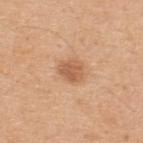The lesion was photographed on a routine skin check and not biopsied; there is no pathology result. Measured at roughly 3 mm in maximum diameter. A 15 mm close-up tile from a total-body photography series done for melanoma screening. Located on the upper back. A male subject roughly 50 years of age. This is a white-light tile.